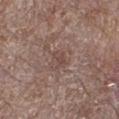Imaged during a routine full-body skin examination; the lesion was not biopsied and no histopathology is available.
A male subject, approximately 70 years of age.
Located on the right lower leg.
Cropped from a whole-body photographic skin survey; the tile spans about 15 mm.